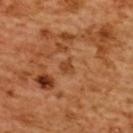Clinical impression:
Recorded during total-body skin imaging; not selected for excision or biopsy.
Context:
From the back. The subject is a female aged 53–57. A lesion tile, about 15 mm wide, cut from a 3D total-body photograph.